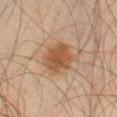Findings:
• location: the leg
• patient: male, in their mid- to late 40s
• image source: ~15 mm tile from a whole-body skin photo
• lesion diameter: about 4 mm